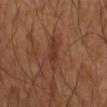No biopsy was performed on this lesion — it was imaged during a full skin examination and was not determined to be concerning.